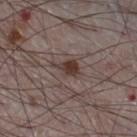Assessment: No biopsy was performed on this lesion — it was imaged during a full skin examination and was not determined to be concerning. Context: The lesion is on the right thigh. The patient is a male aged around 75. A roughly 15 mm field-of-view crop from a total-body skin photograph.